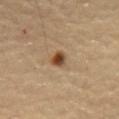Longest diameter approximately 2.5 mm. A roughly 15 mm field-of-view crop from a total-body skin photograph. A male patient aged approximately 55. Located on the abdomen. The tile uses cross-polarized illumination.